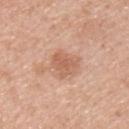Located on the back.
An algorithmic analysis of the crop reported an average lesion color of about L≈60 a*≈22 b*≈32 (CIELAB), a lesion–skin lightness drop of about 9, and a normalized lesion–skin contrast near 6. It also reported a lesion-detection confidence of about 100/100.
A close-up tile cropped from a whole-body skin photograph, about 15 mm across.
Approximately 4 mm at its widest.
A male subject aged around 45.
The tile uses white-light illumination.